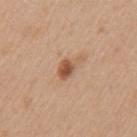Imaged during a routine full-body skin examination; the lesion was not biopsied and no histopathology is available.
The lesion is located on the left upper arm.
Automated tile analysis of the lesion measured a lesion area of about 5 mm², a shape eccentricity near 0.8, and a symmetry-axis asymmetry near 0.3. It also reported a mean CIELAB color near L≈55 a*≈21 b*≈33 and a lesion-to-skin contrast of about 8 (normalized; higher = more distinct). The software also gave a classifier nevus-likeness of about 80/100 and a detector confidence of about 100 out of 100 that the crop contains a lesion.
This is a white-light tile.
A female subject roughly 40 years of age.
A close-up tile cropped from a whole-body skin photograph, about 15 mm across.